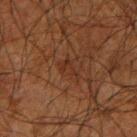follow-up: no biopsy performed (imaged during a skin exam)
image: ~15 mm crop, total-body skin-cancer survey
lesion size: ≈2.5 mm
tile lighting: cross-polarized
patient: male, aged around 65
site: the right upper arm
TBP lesion metrics: a footprint of about 3 mm², a shape eccentricity near 0.85, and a symmetry-axis asymmetry near 0.65; a classifier nevus-likeness of about 0/100